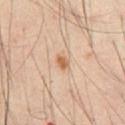No biopsy was performed on this lesion — it was imaged during a full skin examination and was not determined to be concerning. The lesion's longest dimension is about 2 mm. The subject is a male in their mid- to late 60s. The lesion is located on the abdomen. Captured under cross-polarized illumination. Cropped from a whole-body photographic skin survey; the tile spans about 15 mm.